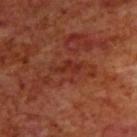Q: Was a biopsy performed?
A: total-body-photography surveillance lesion; no biopsy
Q: Who is the patient?
A: male, in their 70s
Q: What lighting was used for the tile?
A: cross-polarized
Q: What did automated image analysis measure?
A: a footprint of about 6 mm², an eccentricity of roughly 0.85, and a shape-asymmetry score of about 0.45 (0 = symmetric); an average lesion color of about L≈31 a*≈27 b*≈28 (CIELAB) and a lesion-to-skin contrast of about 6 (normalized; higher = more distinct); a within-lesion color-variation index near 2/10 and radial color variation of about 0.5; a classifier nevus-likeness of about 0/100 and a lesion-detection confidence of about 100/100
Q: What is the imaging modality?
A: 15 mm crop, total-body photography
Q: Where on the body is the lesion?
A: the upper back
Q: Lesion size?
A: about 4 mm